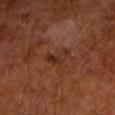Q: Was this lesion biopsied?
A: imaged on a skin check; not biopsied
Q: What is the imaging modality?
A: total-body-photography crop, ~15 mm field of view
Q: What is the anatomic site?
A: the left forearm
Q: What are the patient's age and sex?
A: male, aged around 60